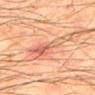Recorded during total-body skin imaging; not selected for excision or biopsy. A male patient aged 63 to 67. Imaged with cross-polarized lighting. Automated tile analysis of the lesion measured a footprint of about 14 mm², an eccentricity of roughly 0.95, and a shape-asymmetry score of about 0.6 (0 = symmetric). The software also gave an average lesion color of about L≈52 a*≈22 b*≈29 (CIELAB), about 8 CIELAB-L* units darker than the surrounding skin, and a normalized border contrast of about 6. The analysis additionally found a border-irregularity index near 7.5/10, internal color variation of about 8 on a 0–10 scale, and peripheral color asymmetry of about 3. It also reported an automated nevus-likeness rating near 5 out of 100. The lesion is on the mid back. Approximately 8 mm at its widest. A region of skin cropped from a whole-body photographic capture, roughly 15 mm wide.